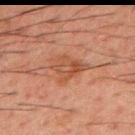Q: Was this lesion biopsied?
A: no biopsy performed (imaged during a skin exam)
Q: What lighting was used for the tile?
A: cross-polarized illumination
Q: Lesion size?
A: ≈4 mm
Q: Automated lesion metrics?
A: a shape eccentricity near 0.65 and a symmetry-axis asymmetry near 0.4; a within-lesion color-variation index near 4.5/10 and a peripheral color-asymmetry measure near 1.5; a nevus-likeness score of about 0/100 and a lesion-detection confidence of about 100/100
Q: Where on the body is the lesion?
A: the mid back
Q: Patient demographics?
A: male, about 50 years old
Q: How was this image acquired?
A: ~15 mm tile from a whole-body skin photo